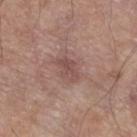{
  "biopsy_status": "not biopsied; imaged during a skin examination",
  "patient": {
    "sex": "male",
    "age_approx": 80
  },
  "lesion_size": {
    "long_diameter_mm_approx": 3.0
  },
  "site": "left lower leg",
  "image": {
    "source": "total-body photography crop",
    "field_of_view_mm": 15
  },
  "lighting": "white-light",
  "automated_metrics": {
    "area_mm2_approx": 4.5
  }
}